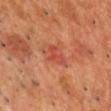This lesion was catalogued during total-body skin photography and was not selected for biopsy. The lesion is located on the chest. Cropped from a total-body skin-imaging series; the visible field is about 15 mm. A male patient aged around 50. The total-body-photography lesion software estimated a mean CIELAB color near L≈50 a*≈34 b*≈36. And it measured a border-irregularity index near 6/10, a color-variation rating of about 2/10, and a peripheral color-asymmetry measure near 0.5. This is a cross-polarized tile.